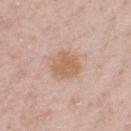Assessment:
This lesion was catalogued during total-body skin photography and was not selected for biopsy.
Image and clinical context:
A 15 mm close-up extracted from a 3D total-body photography capture. A male patient, in their 60s. The lesion is located on the chest.